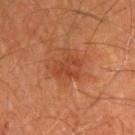Part of a total-body skin-imaging series; this lesion was reviewed on a skin check and was not flagged for biopsy.
A 15 mm close-up extracted from a 3D total-body photography capture.
On the right upper arm.
The subject is a male in their mid- to late 60s.
Imaged with cross-polarized lighting.
Automated image analysis of the tile measured an area of roughly 5.5 mm², an outline eccentricity of about 0.7 (0 = round, 1 = elongated), and a symmetry-axis asymmetry near 0.45. And it measured a border-irregularity index near 6.5/10, a within-lesion color-variation index near 1/10, and a peripheral color-asymmetry measure near 0.5.
Longest diameter approximately 3.5 mm.